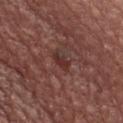The lesion was photographed on a routine skin check and not biopsied; there is no pathology result. A 15 mm close-up tile from a total-body photography series done for melanoma screening. The lesion is located on the chest. The subject is a male roughly 65 years of age. The lesion's longest dimension is about 2.5 mm.